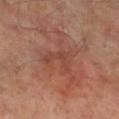This lesion was catalogued during total-body skin photography and was not selected for biopsy. A roughly 15 mm field-of-view crop from a total-body skin photograph. The lesion is on the left leg. A male subject roughly 50 years of age.